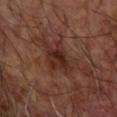biopsy status: catalogued during a skin exam; not biopsied | image source: ~15 mm tile from a whole-body skin photo | body site: the right forearm | patient: male, aged around 65 | tile lighting: cross-polarized | image-analysis metrics: an area of roughly 10 mm², an outline eccentricity of about 0.75 (0 = round, 1 = elongated), and a shape-asymmetry score of about 0.35 (0 = symmetric); a border-irregularity index near 5/10, a within-lesion color-variation index near 3.5/10, and peripheral color asymmetry of about 1; a classifier nevus-likeness of about 0/100 and a detector confidence of about 100 out of 100 that the crop contains a lesion.